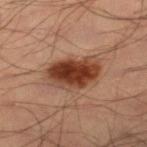Captured during whole-body skin photography for melanoma surveillance; the lesion was not biopsied.